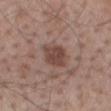Part of a total-body skin-imaging series; this lesion was reviewed on a skin check and was not flagged for biopsy. Located on the mid back. The tile uses white-light illumination. A male subject, about 70 years old. This image is a 15 mm lesion crop taken from a total-body photograph. The recorded lesion diameter is about 3.5 mm. Automated tile analysis of the lesion measured a mean CIELAB color near L≈44 a*≈19 b*≈23, a lesion–skin lightness drop of about 10, and a normalized border contrast of about 8. The analysis additionally found a border-irregularity index near 2/10, internal color variation of about 2.5 on a 0–10 scale, and peripheral color asymmetry of about 1. The analysis additionally found a detector confidence of about 100 out of 100 that the crop contains a lesion.